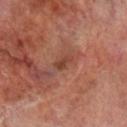Part of a total-body skin-imaging series; this lesion was reviewed on a skin check and was not flagged for biopsy.
Located on the right lower leg.
Longest diameter approximately 2.5 mm.
A male patient, in their 70s.
An algorithmic analysis of the crop reported an average lesion color of about L≈41 a*≈24 b*≈29 (CIELAB) and a normalized border contrast of about 7. And it measured a classifier nevus-likeness of about 0/100 and a detector confidence of about 95 out of 100 that the crop contains a lesion.
A close-up tile cropped from a whole-body skin photograph, about 15 mm across.
Captured under cross-polarized illumination.